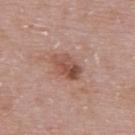{
  "biopsy_status": "not biopsied; imaged during a skin examination",
  "patient": {
    "sex": "female",
    "age_approx": 30
  },
  "automated_metrics": {
    "area_mm2_approx": 7.0,
    "eccentricity": 0.8,
    "shape_asymmetry": 0.2
  },
  "site": "upper back",
  "lesion_size": {
    "long_diameter_mm_approx": 4.0
  },
  "image": {
    "source": "total-body photography crop",
    "field_of_view_mm": 15
  }
}